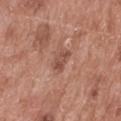notes=no biopsy performed (imaged during a skin exam) | acquisition=~15 mm tile from a whole-body skin photo | automated metrics=a nevus-likeness score of about 0/100 and a lesion-detection confidence of about 100/100 | patient=male, aged 48 to 52 | site=the mid back | size=~3 mm (longest diameter).